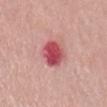The subject is a female aged around 65. Measured at roughly 4 mm in maximum diameter. This is a white-light tile. Automated image analysis of the tile measured a border-irregularity rating of about 1.5/10, a color-variation rating of about 5.5/10, and radial color variation of about 1.5. And it measured an automated nevus-likeness rating near 0 out of 100. On the mid back. A roughly 15 mm field-of-view crop from a total-body skin photograph.